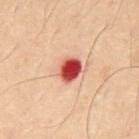Imaged during a routine full-body skin examination; the lesion was not biopsied and no histopathology is available. On the abdomen. A male subject, roughly 50 years of age. This image is a 15 mm lesion crop taken from a total-body photograph.